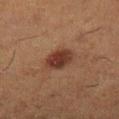Impression:
The lesion was photographed on a routine skin check and not biopsied; there is no pathology result.
Acquisition and patient details:
On the leg. Cropped from a whole-body photographic skin survey; the tile spans about 15 mm. The subject is a female aged around 50.